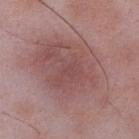Clinical impression: No biopsy was performed on this lesion — it was imaged during a full skin examination and was not determined to be concerning. Context: Automated tile analysis of the lesion measured a lesion area of about 50 mm², an eccentricity of roughly 0.7, and a shape-asymmetry score of about 0.2 (0 = symmetric). It also reported roughly 8 lightness units darker than nearby skin and a lesion-to-skin contrast of about 5.5 (normalized; higher = more distinct). And it measured internal color variation of about 4 on a 0–10 scale. The software also gave a classifier nevus-likeness of about 35/100 and a lesion-detection confidence of about 100/100. A close-up tile cropped from a whole-body skin photograph, about 15 mm across. A male patient, aged around 50. The recorded lesion diameter is about 10.5 mm. Imaged with white-light lighting. On the right lower leg.